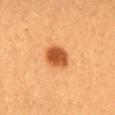The lesion was photographed on a routine skin check and not biopsied; there is no pathology result.
Measured at roughly 3 mm in maximum diameter.
A 15 mm crop from a total-body photograph taken for skin-cancer surveillance.
The subject is a female roughly 30 years of age.
From the chest.